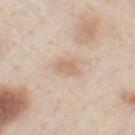This lesion was catalogued during total-body skin photography and was not selected for biopsy. A male patient in their 60s. Cropped from a whole-body photographic skin survey; the tile spans about 15 mm. The lesion is located on the chest.